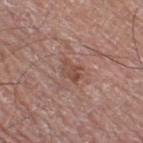Imaged during a routine full-body skin examination; the lesion was not biopsied and no histopathology is available. The recorded lesion diameter is about 2.5 mm. From the right thigh. The total-body-photography lesion software estimated a mean CIELAB color near L≈48 a*≈21 b*≈25, a lesion–skin lightness drop of about 8, and a normalized lesion–skin contrast near 6.5. This is a white-light tile. A close-up tile cropped from a whole-body skin photograph, about 15 mm across. A male subject, in their mid- to late 70s.